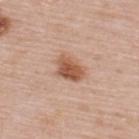Findings:
* biopsy status: catalogued during a skin exam; not biopsied
* patient: female, aged around 50
* image: ~15 mm tile from a whole-body skin photo
* location: the upper back